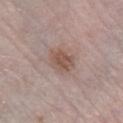{
  "biopsy_status": "not biopsied; imaged during a skin examination",
  "site": "right lower leg",
  "image": {
    "source": "total-body photography crop",
    "field_of_view_mm": 15
  },
  "patient": {
    "sex": "female",
    "age_approx": 75
  }
}